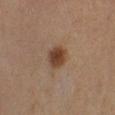Captured during whole-body skin photography for melanoma surveillance; the lesion was not biopsied. Located on the right lower leg. Cropped from a total-body skin-imaging series; the visible field is about 15 mm. The subject is a female approximately 55 years of age.